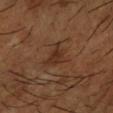image source: ~15 mm crop, total-body skin-cancer survey | automated metrics: a footprint of about 5.5 mm², an eccentricity of roughly 0.75, and a shape-asymmetry score of about 0.45 (0 = symmetric); a lesion color around L≈31 a*≈19 b*≈28 in CIELAB and a normalized lesion–skin contrast near 6.5; a lesion-detection confidence of about 100/100 | subject: male, roughly 65 years of age | illumination: cross-polarized illumination | body site: the right forearm.